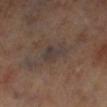workup=no biopsy performed (imaged during a skin exam)
acquisition=~15 mm tile from a whole-body skin photo
location=the right lower leg